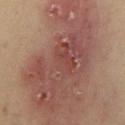follow-up — catalogued during a skin exam; not biopsied | patient — male, aged approximately 55 | imaging modality — total-body-photography crop, ~15 mm field of view | anatomic site — the mid back.